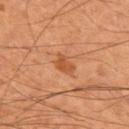Assessment:
Imaged during a routine full-body skin examination; the lesion was not biopsied and no histopathology is available.
Context:
Cropped from a total-body skin-imaging series; the visible field is about 15 mm. The lesion's longest dimension is about 3 mm. The subject is a male in their mid- to late 50s. An algorithmic analysis of the crop reported a footprint of about 4 mm², an outline eccentricity of about 0.75 (0 = round, 1 = elongated), and two-axis asymmetry of about 0.4. The analysis additionally found a lesion color around L≈51 a*≈26 b*≈38 in CIELAB, about 9 CIELAB-L* units darker than the surrounding skin, and a lesion-to-skin contrast of about 6.5 (normalized; higher = more distinct). Imaged with cross-polarized lighting. From the upper back.